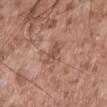Impression:
Imaged during a routine full-body skin examination; the lesion was not biopsied and no histopathology is available.
Clinical summary:
Cropped from a total-body skin-imaging series; the visible field is about 15 mm. Automated tile analysis of the lesion measured a footprint of about 3.5 mm² and an eccentricity of roughly 0.8. And it measured an average lesion color of about L≈51 a*≈21 b*≈27 (CIELAB), roughly 9 lightness units darker than nearby skin, and a lesion-to-skin contrast of about 6 (normalized; higher = more distinct). A male patient aged approximately 55. The tile uses white-light illumination. From the mid back.